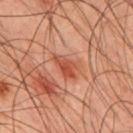| field | value |
|---|---|
| workup | total-body-photography surveillance lesion; no biopsy |
| tile lighting | cross-polarized |
| patient | male, in their mid-40s |
| acquisition | ~15 mm crop, total-body skin-cancer survey |
| lesion diameter | ~3.5 mm (longest diameter) |
| automated lesion analysis | a lesion area of about 5 mm², an eccentricity of roughly 0.85, and a symmetry-axis asymmetry near 0.3; border irregularity of about 3.5 on a 0–10 scale, a color-variation rating of about 3.5/10, and radial color variation of about 1.5 |
| body site | the mid back |